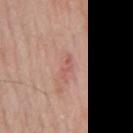Clinical impression:
This lesion was catalogued during total-body skin photography and was not selected for biopsy.